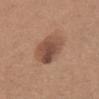Captured during whole-body skin photography for melanoma surveillance; the lesion was not biopsied. A female patient, roughly 45 years of age. A lesion tile, about 15 mm wide, cut from a 3D total-body photograph. The lesion is on the abdomen. The tile uses white-light illumination. About 4.5 mm across.